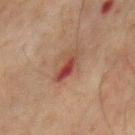The lesion was photographed on a routine skin check and not biopsied; there is no pathology result.
Cropped from a total-body skin-imaging series; the visible field is about 15 mm.
Imaged with cross-polarized lighting.
The patient is a male about 65 years old.
From the front of the torso.
Approximately 3.5 mm at its widest.